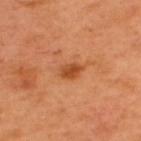site: the upper back | imaging modality: 15 mm crop, total-body photography | patient: male, approximately 50 years of age.